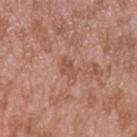Notes:
* workup · imaged on a skin check; not biopsied
* body site · the left upper arm
* subject · male, aged 38–42
* image source · ~15 mm tile from a whole-body skin photo